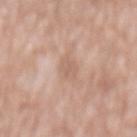Imaged during a routine full-body skin examination; the lesion was not biopsied and no histopathology is available. A male subject, in their 60s. On the abdomen. Imaged with white-light lighting. The total-body-photography lesion software estimated a nevus-likeness score of about 0/100 and a lesion-detection confidence of about 100/100. The lesion's longest dimension is about 4 mm. This image is a 15 mm lesion crop taken from a total-body photograph.